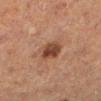{"biopsy_status": "not biopsied; imaged during a skin examination", "site": "left lower leg", "automated_metrics": {"eccentricity": 0.35, "shape_asymmetry": 0.2, "border_irregularity_0_10": 2.0, "color_variation_0_10": 4.0, "peripheral_color_asymmetry": 1.5, "lesion_detection_confidence_0_100": 100}, "image": {"source": "total-body photography crop", "field_of_view_mm": 15}, "patient": {"sex": "female", "age_approx": 40}}